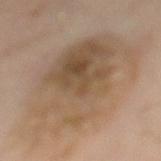Context: The lesion is located on the mid back. The subject is in their mid-50s. Cropped from a whole-body photographic skin survey; the tile spans about 15 mm. The lesion-visualizer software estimated an outline eccentricity of about 0.75 (0 = round, 1 = elongated) and two-axis asymmetry of about 0.25. It also reported a border-irregularity rating of about 5/10, a within-lesion color-variation index near 7/10, and peripheral color asymmetry of about 2.5. It also reported an automated nevus-likeness rating near 5 out of 100 and a detector confidence of about 100 out of 100 that the crop contains a lesion. Imaged with cross-polarized lighting. Approximately 13 mm at its widest.